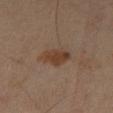Assessment:
The lesion was tiled from a total-body skin photograph and was not biopsied.
Clinical summary:
Imaged with cross-polarized lighting. On the left thigh. An algorithmic analysis of the crop reported a mean CIELAB color near L≈37 a*≈16 b*≈27 and roughly 8 lightness units darker than nearby skin. The analysis additionally found border irregularity of about 2.5 on a 0–10 scale and peripheral color asymmetry of about 1. The analysis additionally found a nevus-likeness score of about 40/100 and a lesion-detection confidence of about 100/100. A male patient, about 65 years old. A region of skin cropped from a whole-body photographic capture, roughly 15 mm wide.